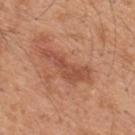<tbp_lesion>
  <biopsy_status>not biopsied; imaged during a skin examination</biopsy_status>
  <lesion_size>
    <long_diameter_mm_approx>6.0</long_diameter_mm_approx>
  </lesion_size>
  <image>
    <source>total-body photography crop</source>
    <field_of_view_mm>15</field_of_view_mm>
  </image>
  <site>upper back</site>
  <patient>
    <sex>male</sex>
    <age_approx>45</age_approx>
  </patient>
  <automated_metrics>
    <cielab_L>51</cielab_L>
    <cielab_a>26</cielab_a>
    <cielab_b>32</cielab_b>
    <vs_skin_darker_L>9.0</vs_skin_darker_L>
    <vs_skin_contrast_norm>6.5</vs_skin_contrast_norm>
  </automated_metrics>
  <lighting>white-light</lighting>
</tbp_lesion>